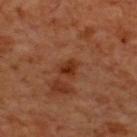The lesion was photographed on a routine skin check and not biopsied; there is no pathology result.
On the upper back.
The patient is a male aged 68–72.
A roughly 15 mm field-of-view crop from a total-body skin photograph.